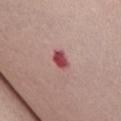biopsy status = total-body-photography surveillance lesion; no biopsy | subject = female, in their mid-60s | body site = the abdomen | imaging modality = total-body-photography crop, ~15 mm field of view | lesion diameter = ≈2.5 mm.